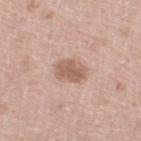Notes:
– follow-up: total-body-photography surveillance lesion; no biopsy
– lesion size: about 3.5 mm
– site: the left lower leg
– illumination: white-light illumination
– imaging modality: ~15 mm crop, total-body skin-cancer survey
– subject: male, aged 63–67